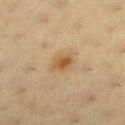The lesion-visualizer software estimated an area of roughly 5.5 mm². It also reported an average lesion color of about L≈48 a*≈15 b*≈33 (CIELAB), about 8 CIELAB-L* units darker than the surrounding skin, and a normalized border contrast of about 7.5. It also reported a color-variation rating of about 3.5/10 and peripheral color asymmetry of about 1. The software also gave an automated nevus-likeness rating near 90 out of 100 and a detector confidence of about 100 out of 100 that the crop contains a lesion. A roughly 15 mm field-of-view crop from a total-body skin photograph. Measured at roughly 3 mm in maximum diameter. Located on the leg. The tile uses cross-polarized illumination. The subject is a female aged approximately 40.